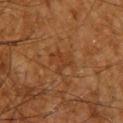This lesion was catalogued during total-body skin photography and was not selected for biopsy. Approximately 3 mm at its widest. A lesion tile, about 15 mm wide, cut from a 3D total-body photograph. An algorithmic analysis of the crop reported border irregularity of about 7.5 on a 0–10 scale, internal color variation of about 0 on a 0–10 scale, and peripheral color asymmetry of about 0. It also reported a classifier nevus-likeness of about 0/100 and a lesion-detection confidence of about 100/100. On the upper back. A male subject aged around 60.